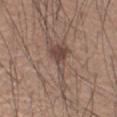Q: Was this lesion biopsied?
A: imaged on a skin check; not biopsied